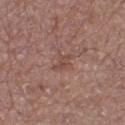Assessment:
This lesion was catalogued during total-body skin photography and was not selected for biopsy.
Acquisition and patient details:
Longest diameter approximately 2.5 mm. A region of skin cropped from a whole-body photographic capture, roughly 15 mm wide. On the right lower leg. Automated tile analysis of the lesion measured a mean CIELAB color near L≈46 a*≈21 b*≈24, roughly 7 lightness units darker than nearby skin, and a lesion-to-skin contrast of about 5.5 (normalized; higher = more distinct). And it measured border irregularity of about 4 on a 0–10 scale and a color-variation rating of about 0.5/10. The software also gave a classifier nevus-likeness of about 0/100 and a detector confidence of about 100 out of 100 that the crop contains a lesion. Imaged with white-light lighting. The subject is a male aged 63 to 67.